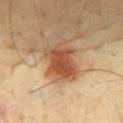{"biopsy_status": "not biopsied; imaged during a skin examination", "site": "mid back", "lighting": "cross-polarized", "patient": {"sex": "male", "age_approx": 55}, "image": {"source": "total-body photography crop", "field_of_view_mm": 15}}